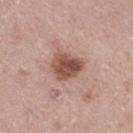| feature | finding |
|---|---|
| workup | no biopsy performed (imaged during a skin exam) |
| image | ~15 mm tile from a whole-body skin photo |
| anatomic site | the leg |
| subject | male, in their mid-70s |
| TBP lesion metrics | a footprint of about 9.5 mm² and an outline eccentricity of about 0.6 (0 = round, 1 = elongated); an average lesion color of about L≈51 a*≈21 b*≈27 (CIELAB), roughly 14 lightness units darker than nearby skin, and a normalized lesion–skin contrast near 9.5 |
| illumination | white-light illumination |
| lesion diameter | about 4 mm |